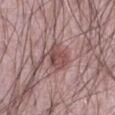| feature | finding |
|---|---|
| notes | total-body-photography surveillance lesion; no biopsy |
| acquisition | total-body-photography crop, ~15 mm field of view |
| subject | male, aged approximately 65 |
| location | the abdomen |